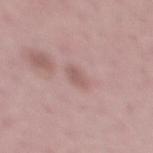No biopsy was performed on this lesion — it was imaged during a full skin examination and was not determined to be concerning. This is a white-light tile. From the mid back. Cropped from a whole-body photographic skin survey; the tile spans about 15 mm. A male patient approximately 50 years of age.